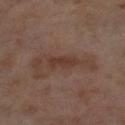About 3.5 mm across. A female subject in their mid- to late 50s. From the right thigh. A 15 mm crop from a total-body photograph taken for skin-cancer surveillance.